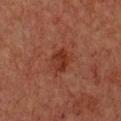follow-up: imaged on a skin check; not biopsied
location: the chest
acquisition: ~15 mm crop, total-body skin-cancer survey
automated metrics: a lesion color around L≈28 a*≈24 b*≈27 in CIELAB, a lesion–skin lightness drop of about 7, and a lesion-to-skin contrast of about 8 (normalized; higher = more distinct); a nevus-likeness score of about 15/100
size: about 3 mm
patient: female, roughly 50 years of age
tile lighting: cross-polarized illumination